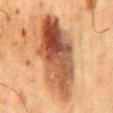illumination: cross-polarized
size: ≈11 mm
imaging modality: total-body-photography crop, ~15 mm field of view
subject: male, aged around 65
anatomic site: the mid back
automated metrics: a footprint of about 42 mm², a shape eccentricity near 0.9, and a shape-asymmetry score of about 0.2 (0 = symmetric); a classifier nevus-likeness of about 15/100 and a lesion-detection confidence of about 100/100